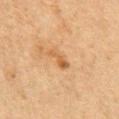workup: imaged on a skin check; not biopsied | automated metrics: a lesion–skin lightness drop of about 8; a nevus-likeness score of about 5/100 and lesion-presence confidence of about 100/100 | illumination: cross-polarized | image source: ~15 mm tile from a whole-body skin photo | location: the chest | patient: male, in their mid- to late 70s | lesion diameter: ≈3.5 mm.